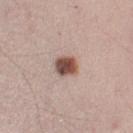Clinical impression: Imaged during a routine full-body skin examination; the lesion was not biopsied and no histopathology is available. Acquisition and patient details: A male patient aged 33–37. The lesion's longest dimension is about 2.5 mm. The lesion is on the left upper arm. Imaged with white-light lighting. A region of skin cropped from a whole-body photographic capture, roughly 15 mm wide.